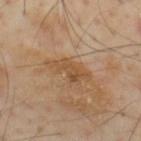Clinical impression: Recorded during total-body skin imaging; not selected for excision or biopsy. Acquisition and patient details: Automated image analysis of the tile measured an average lesion color of about L≈51 a*≈18 b*≈35 (CIELAB), roughly 8 lightness units darker than nearby skin, and a normalized lesion–skin contrast near 7. And it measured border irregularity of about 6 on a 0–10 scale, internal color variation of about 3.5 on a 0–10 scale, and a peripheral color-asymmetry measure near 1. The analysis additionally found a classifier nevus-likeness of about 0/100 and a lesion-detection confidence of about 100/100. Longest diameter approximately 5 mm. A close-up tile cropped from a whole-body skin photograph, about 15 mm across. The patient is a male roughly 55 years of age. On the mid back. Captured under cross-polarized illumination.